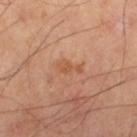<record>
  <biopsy_status>not biopsied; imaged during a skin examination</biopsy_status>
  <lighting>cross-polarized</lighting>
  <image>
    <source>total-body photography crop</source>
    <field_of_view_mm>15</field_of_view_mm>
  </image>
  <patient>
    <sex>male</sex>
    <age_approx>65</age_approx>
  </patient>
  <lesion_size>
    <long_diameter_mm_approx>3.0</long_diameter_mm_approx>
  </lesion_size>
  <site>left thigh</site>
</record>